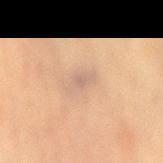follow-up: total-body-photography surveillance lesion; no biopsy | image source: 15 mm crop, total-body photography | subject: female, approximately 65 years of age | site: the mid back.